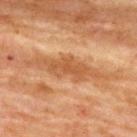This lesion was catalogued during total-body skin photography and was not selected for biopsy. Imaged with cross-polarized lighting. A 15 mm close-up tile from a total-body photography series done for melanoma screening. A female patient approximately 80 years of age. Automated tile analysis of the lesion measured border irregularity of about 4.5 on a 0–10 scale, a within-lesion color-variation index near 1.5/10, and peripheral color asymmetry of about 0.5. The lesion is on the upper back.